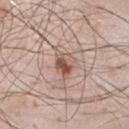Findings:
• biopsy status: catalogued during a skin exam; not biopsied
• subject: male, aged approximately 55
• acquisition: ~15 mm crop, total-body skin-cancer survey
• site: the chest
• lighting: white-light
• size: about 3 mm